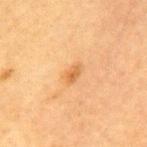  biopsy_status: not biopsied; imaged during a skin examination
  lighting: cross-polarized
  lesion_size:
    long_diameter_mm_approx: 2.5
  image:
    source: total-body photography crop
    field_of_view_mm: 15
  patient:
    sex: female
    age_approx: 60
  site: mid back